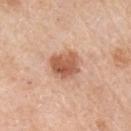Recorded during total-body skin imaging; not selected for excision or biopsy.
On the right upper arm.
This is a white-light tile.
The subject is a male in their 60s.
A region of skin cropped from a whole-body photographic capture, roughly 15 mm wide.
Measured at roughly 3.5 mm in maximum diameter.